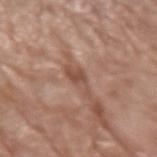Q: Was a biopsy performed?
A: catalogued during a skin exam; not biopsied
Q: What did automated image analysis measure?
A: a footprint of about 4.5 mm² and an eccentricity of roughly 0.9; a mean CIELAB color near L≈50 a*≈21 b*≈28 and a lesion–skin lightness drop of about 9; a nevus-likeness score of about 0/100 and lesion-presence confidence of about 75/100
Q: What is the anatomic site?
A: the left upper arm
Q: What is the imaging modality?
A: ~15 mm crop, total-body skin-cancer survey
Q: Patient demographics?
A: male, aged 78–82
Q: Illumination type?
A: white-light illumination